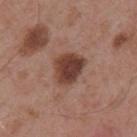Notes:
- patient: male, about 55 years old
- body site: the mid back
- automated lesion analysis: a footprint of about 10 mm², an outline eccentricity of about 0.55 (0 = round, 1 = elongated), and a shape-asymmetry score of about 0.25 (0 = symmetric); lesion-presence confidence of about 100/100
- size: about 4 mm
- acquisition: 15 mm crop, total-body photography